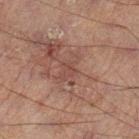Assessment: No biopsy was performed on this lesion — it was imaged during a full skin examination and was not determined to be concerning. Acquisition and patient details: Cropped from a whole-body photographic skin survey; the tile spans about 15 mm. This is a cross-polarized tile. The total-body-photography lesion software estimated a footprint of about 5.5 mm² and a shape eccentricity near 0.8. It also reported a lesion-to-skin contrast of about 4.5 (normalized; higher = more distinct). And it measured a border-irregularity rating of about 5/10, a within-lesion color-variation index near 1.5/10, and peripheral color asymmetry of about 0.5. Located on the left lower leg. A male patient approximately 60 years of age. Longest diameter approximately 3.5 mm.